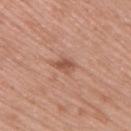Part of a total-body skin-imaging series; this lesion was reviewed on a skin check and was not flagged for biopsy. A male subject, approximately 60 years of age. Captured under white-light illumination. A roughly 15 mm field-of-view crop from a total-body skin photograph. The lesion is on the right upper arm. An algorithmic analysis of the crop reported a footprint of about 3.5 mm², an eccentricity of roughly 0.75, and a shape-asymmetry score of about 0.3 (0 = symmetric). And it measured an average lesion color of about L≈53 a*≈23 b*≈30 (CIELAB), roughly 10 lightness units darker than nearby skin, and a normalized lesion–skin contrast near 7. It also reported a nevus-likeness score of about 50/100 and lesion-presence confidence of about 100/100. About 2.5 mm across.